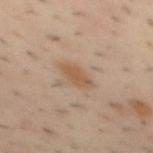workup = imaged on a skin check; not biopsied | subject = male, approximately 40 years of age | size = about 3.5 mm | imaging modality = total-body-photography crop, ~15 mm field of view | illumination = cross-polarized | anatomic site = the back.